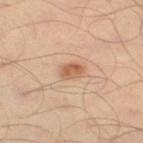Recorded during total-body skin imaging; not selected for excision or biopsy. The subject is a male aged 38–42. Cropped from a total-body skin-imaging series; the visible field is about 15 mm. Longest diameter approximately 2.5 mm. Imaged with cross-polarized lighting. From the left thigh. Automated tile analysis of the lesion measured a footprint of about 4 mm², an outline eccentricity of about 0.65 (0 = round, 1 = elongated), and two-axis asymmetry of about 0.15. The software also gave a lesion–skin lightness drop of about 11 and a lesion-to-skin contrast of about 8 (normalized; higher = more distinct). The analysis additionally found a lesion-detection confidence of about 100/100.